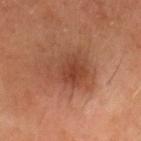Q: Was this lesion biopsied?
A: imaged on a skin check; not biopsied
Q: Lesion location?
A: the head or neck
Q: What is the imaging modality?
A: total-body-photography crop, ~15 mm field of view
Q: What are the patient's age and sex?
A: male, aged 48 to 52